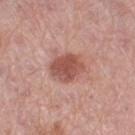• follow-up — total-body-photography surveillance lesion; no biopsy
• size — ~4 mm (longest diameter)
• lighting — white-light illumination
• image source — total-body-photography crop, ~15 mm field of view
• site — the right thigh
• patient — female, about 45 years old